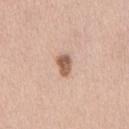Imaged with white-light lighting.
Cropped from a total-body skin-imaging series; the visible field is about 15 mm.
The lesion's longest dimension is about 2.5 mm.
On the chest.
A male subject aged 38 to 42.